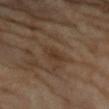• subject: female, approximately 70 years of age
• anatomic site: the left forearm
• image source: ~15 mm crop, total-body skin-cancer survey
• lesion diameter: ~2.5 mm (longest diameter)
• image-analysis metrics: a shape eccentricity near 0.8; a border-irregularity index near 2.5/10, a color-variation rating of about 1.5/10, and radial color variation of about 0.5
• illumination: cross-polarized